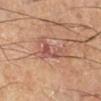| key | value |
|---|---|
| biopsy status | imaged on a skin check; not biopsied |
| acquisition | total-body-photography crop, ~15 mm field of view |
| lesion diameter | about 5.5 mm |
| automated lesion analysis | an average lesion color of about L≈45 a*≈20 b*≈23 (CIELAB), roughly 7 lightness units darker than nearby skin, and a normalized border contrast of about 6.5; a border-irregularity rating of about 6/10, internal color variation of about 5.5 on a 0–10 scale, and a peripheral color-asymmetry measure near 2; lesion-presence confidence of about 85/100 |
| tile lighting | cross-polarized |
| subject | male, approximately 65 years of age |
| site | the left lower leg |